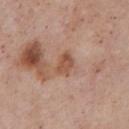follow-up: imaged on a skin check; not biopsied | image: total-body-photography crop, ~15 mm field of view | tile lighting: white-light illumination | patient: male, roughly 55 years of age | TBP lesion metrics: a mean CIELAB color near L≈53 a*≈21 b*≈30, roughly 9 lightness units darker than nearby skin, and a normalized lesion–skin contrast near 7; a nevus-likeness score of about 0/100 | location: the chest | diameter: ~3 mm (longest diameter).